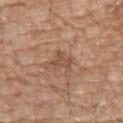Clinical impression:
No biopsy was performed on this lesion — it was imaged during a full skin examination and was not determined to be concerning.
Context:
Captured under white-light illumination. A 15 mm crop from a total-body photograph taken for skin-cancer surveillance. A male subject, approximately 80 years of age. From the left upper arm. An algorithmic analysis of the crop reported an area of roughly 3.5 mm², a shape eccentricity near 0.7, and a shape-asymmetry score of about 0.4 (0 = symmetric). It also reported a border-irregularity index near 4.5/10, a within-lesion color-variation index near 1/10, and radial color variation of about 0.5.